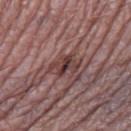Captured during whole-body skin photography for melanoma surveillance; the lesion was not biopsied. The lesion is on the right thigh. A female subject, aged 68–72. The lesion-visualizer software estimated an outline eccentricity of about 0.75 (0 = round, 1 = elongated) and a shape-asymmetry score of about 0.3 (0 = symmetric). The software also gave an average lesion color of about L≈38 a*≈20 b*≈19 (CIELAB), roughly 11 lightness units darker than nearby skin, and a lesion-to-skin contrast of about 9 (normalized; higher = more distinct). Imaged with white-light lighting. The recorded lesion diameter is about 3.5 mm. Cropped from a total-body skin-imaging series; the visible field is about 15 mm.